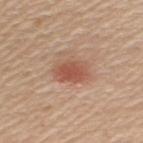Q: Was a biopsy performed?
A: catalogued during a skin exam; not biopsied
Q: What is the anatomic site?
A: the upper back
Q: What are the patient's age and sex?
A: female, aged 48 to 52
Q: What kind of image is this?
A: total-body-photography crop, ~15 mm field of view
Q: Lesion size?
A: about 4 mm